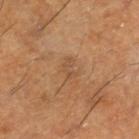The lesion was photographed on a routine skin check and not biopsied; there is no pathology result.
A roughly 15 mm field-of-view crop from a total-body skin photograph.
A male subject, aged approximately 60.
The lesion is located on the right lower leg.
The lesion's longest dimension is about 2.5 mm.
The total-body-photography lesion software estimated an eccentricity of roughly 0.8 and a shape-asymmetry score of about 0.4 (0 = symmetric). And it measured border irregularity of about 4 on a 0–10 scale, internal color variation of about 1 on a 0–10 scale, and peripheral color asymmetry of about 0.5. The analysis additionally found an automated nevus-likeness rating near 0 out of 100 and a detector confidence of about 100 out of 100 that the crop contains a lesion.